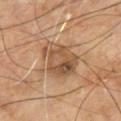{
  "biopsy_status": "not biopsied; imaged during a skin examination"
}